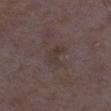  biopsy_status: not biopsied; imaged during a skin examination
  automated_metrics:
    eccentricity: 0.8
    shape_asymmetry: 0.4
    cielab_L: 34
    cielab_a: 12
    cielab_b: 17
    vs_skin_darker_L: 5.0
    lesion_detection_confidence_0_100: 100
  patient:
    sex: female
    age_approx: 35
  site: left lower leg
  image:
    source: total-body photography crop
    field_of_view_mm: 15
  lighting: white-light